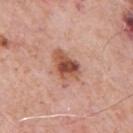subject = male, in their 80s | imaging modality = ~15 mm crop, total-body skin-cancer survey | location = the chest | illumination = white-light | size = ≈4.5 mm.